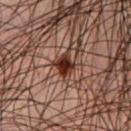Assessment:
The lesion was tiled from a total-body skin photograph and was not biopsied.
Background:
A 15 mm crop from a total-body photograph taken for skin-cancer surveillance. A male subject roughly 45 years of age. The recorded lesion diameter is about 2.5 mm. The lesion is on the chest. The tile uses cross-polarized illumination.